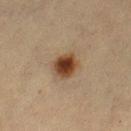Findings:
* imaging modality: 15 mm crop, total-body photography
* lighting: cross-polarized illumination
* body site: the leg
* diameter: ~3.5 mm (longest diameter)
* subject: female, roughly 55 years of age
* automated metrics: an area of roughly 7 mm², an outline eccentricity of about 0.6 (0 = round, 1 = elongated), and a symmetry-axis asymmetry near 0.2